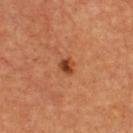Q: Who is the patient?
A: male, in their mid-60s
Q: What is the imaging modality?
A: ~15 mm tile from a whole-body skin photo
Q: What is the anatomic site?
A: the chest